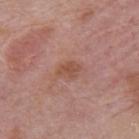Recorded during total-body skin imaging; not selected for excision or biopsy.
Located on the mid back.
Measured at roughly 2.5 mm in maximum diameter.
Cropped from a whole-body photographic skin survey; the tile spans about 15 mm.
The subject is a male aged around 75.